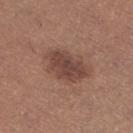Case summary:
– biopsy status: imaged on a skin check; not biopsied
– anatomic site: the right lower leg
– patient: female, about 35 years old
– illumination: white-light
– acquisition: total-body-photography crop, ~15 mm field of view
– size: ≈5.5 mm
– TBP lesion metrics: a lesion area of about 15 mm², an outline eccentricity of about 0.75 (0 = round, 1 = elongated), and a shape-asymmetry score of about 0.2 (0 = symmetric); border irregularity of about 3 on a 0–10 scale and internal color variation of about 3.5 on a 0–10 scale